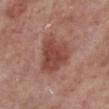This lesion was catalogued during total-body skin photography and was not selected for biopsy.
A male patient, approximately 70 years of age.
Imaged with white-light lighting.
On the right lower leg.
A close-up tile cropped from a whole-body skin photograph, about 15 mm across.